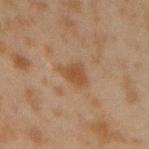biopsy status: imaged on a skin check; not biopsied | illumination: cross-polarized | subject: male, aged approximately 45 | anatomic site: the right forearm | automated metrics: a footprint of about 5.5 mm², an eccentricity of roughly 0.65, and two-axis asymmetry of about 0.35; a mean CIELAB color near L≈39 a*≈17 b*≈28, roughly 7 lightness units darker than nearby skin, and a lesion-to-skin contrast of about 7 (normalized; higher = more distinct); a border-irregularity rating of about 3.5/10 and a peripheral color-asymmetry measure near 0.5 | lesion diameter: about 3.5 mm | acquisition: 15 mm crop, total-body photography.